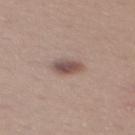Impression: The lesion was photographed on a routine skin check and not biopsied; there is no pathology result. Context: A female patient in their mid- to late 20s. The total-body-photography lesion software estimated a lesion area of about 5.5 mm² and a shape-asymmetry score of about 0.2 (0 = symmetric). The software also gave an average lesion color of about L≈51 a*≈17 b*≈21 (CIELAB) and about 12 CIELAB-L* units darker than the surrounding skin. The software also gave a detector confidence of about 100 out of 100 that the crop contains a lesion. The lesion's longest dimension is about 3 mm. From the mid back. The tile uses white-light illumination. Cropped from a total-body skin-imaging series; the visible field is about 15 mm.